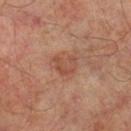Captured during whole-body skin photography for melanoma surveillance; the lesion was not biopsied. A 15 mm close-up extracted from a 3D total-body photography capture. The subject is a male roughly 50 years of age. Captured under cross-polarized illumination. From the left leg. An algorithmic analysis of the crop reported a classifier nevus-likeness of about 10/100 and lesion-presence confidence of about 100/100. The recorded lesion diameter is about 3 mm.